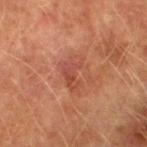| key | value |
|---|---|
| biopsy status | catalogued during a skin exam; not biopsied |
| image | ~15 mm crop, total-body skin-cancer survey |
| subject | male, aged approximately 75 |
| location | the leg |